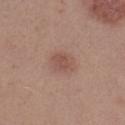The lesion was tiled from a total-body skin photograph and was not biopsied. The tile uses white-light illumination. Cropped from a whole-body photographic skin survey; the tile spans about 15 mm. On the left thigh. The lesion's longest dimension is about 2.5 mm. A female patient aged 28 to 32.